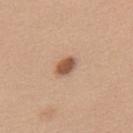{"biopsy_status": "not biopsied; imaged during a skin examination", "automated_metrics": {"vs_skin_darker_L": 14.0, "vs_skin_contrast_norm": 9.0, "border_irregularity_0_10": 1.0, "color_variation_0_10": 3.0, "peripheral_color_asymmetry": 1.0, "nevus_likeness_0_100": 100, "lesion_detection_confidence_0_100": 100}, "site": "upper back", "lighting": "white-light", "image": {"source": "total-body photography crop", "field_of_view_mm": 15}, "patient": {"sex": "female", "age_approx": 40}, "lesion_size": {"long_diameter_mm_approx": 3.0}}